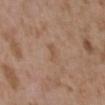biopsy_status: not biopsied; imaged during a skin examination
lighting: white-light
site: upper back
patient:
  sex: female
  age_approx: 35
image:
  source: total-body photography crop
  field_of_view_mm: 15